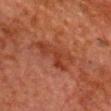biopsy status: imaged on a skin check; not biopsied
imaging modality: ~15 mm crop, total-body skin-cancer survey
illumination: cross-polarized illumination
site: the chest
image-analysis metrics: a border-irregularity rating of about 5/10, internal color variation of about 3.5 on a 0–10 scale, and peripheral color asymmetry of about 1
patient: male, in their 80s
lesion diameter: about 6.5 mm